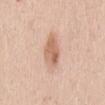Notes:
• follow-up: total-body-photography surveillance lesion; no biopsy
• size: ~3.5 mm (longest diameter)
• imaging modality: 15 mm crop, total-body photography
• tile lighting: white-light
• patient: female, aged 38–42
• TBP lesion metrics: a footprint of about 7.5 mm², a shape eccentricity near 0.65, and a symmetry-axis asymmetry near 0.2; a lesion color around L≈64 a*≈21 b*≈31 in CIELAB and about 11 CIELAB-L* units darker than the surrounding skin; a detector confidence of about 100 out of 100 that the crop contains a lesion
• site: the mid back